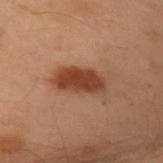This lesion was catalogued during total-body skin photography and was not selected for biopsy. A 15 mm close-up extracted from a 3D total-body photography capture. The patient is a female aged 53 to 57. The lesion is located on the left arm.